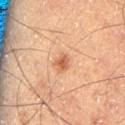Q: Was this lesion biopsied?
A: catalogued during a skin exam; not biopsied
Q: What is the anatomic site?
A: the left thigh
Q: Who is the patient?
A: male, approximately 60 years of age
Q: How was this image acquired?
A: ~15 mm crop, total-body skin-cancer survey
Q: What lighting was used for the tile?
A: cross-polarized
Q: How large is the lesion?
A: ≈2 mm